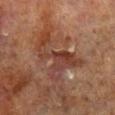Findings:
* biopsy status: total-body-photography surveillance lesion; no biopsy
* diameter: about 7.5 mm
* acquisition: 15 mm crop, total-body photography
* location: the right lower leg
* patient: male, about 70 years old
* illumination: cross-polarized illumination
* automated lesion analysis: an outline eccentricity of about 0.85 (0 = round, 1 = elongated) and a shape-asymmetry score of about 0.75 (0 = symmetric); a nevus-likeness score of about 0/100 and lesion-presence confidence of about 65/100